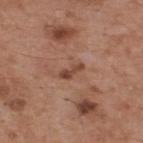Captured during whole-body skin photography for melanoma surveillance; the lesion was not biopsied. Captured under white-light illumination. This image is a 15 mm lesion crop taken from a total-body photograph. The lesion is located on the back. The subject is a male in their mid-50s.